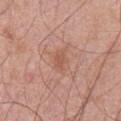{
  "biopsy_status": "not biopsied; imaged during a skin examination",
  "patient": {
    "sex": "male",
    "age_approx": 70
  },
  "lighting": "white-light",
  "site": "front of the torso",
  "image": {
    "source": "total-body photography crop",
    "field_of_view_mm": 15
  },
  "lesion_size": {
    "long_diameter_mm_approx": 2.5
  }
}